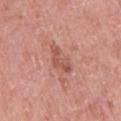  biopsy_status: not biopsied; imaged during a skin examination
  automated_metrics:
    area_mm2_approx: 5.5
    eccentricity: 0.9
    shape_asymmetry: 0.45
    border_irregularity_0_10: 5.0
    peripheral_color_asymmetry: 0.5
  site: right upper arm
  patient:
    sex: female
    age_approx: 50
  image:
    source: total-body photography crop
    field_of_view_mm: 15